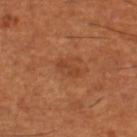<case>
<biopsy_status>not biopsied; imaged during a skin examination</biopsy_status>
</case>